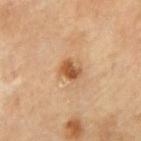Findings:
• follow-up — imaged on a skin check; not biopsied
• tile lighting — cross-polarized
• patient — male, roughly 70 years of age
• image source — total-body-photography crop, ~15 mm field of view
• diameter — ≈2.5 mm
• body site — the back